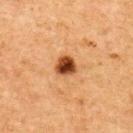{"biopsy_status": "not biopsied; imaged during a skin examination", "patient": {"sex": "female", "age_approx": 60}, "lighting": "cross-polarized", "site": "upper back", "image": {"source": "total-body photography crop", "field_of_view_mm": 15}, "lesion_size": {"long_diameter_mm_approx": 2.5}}